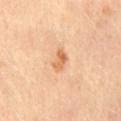  biopsy_status: not biopsied; imaged during a skin examination
  image:
    source: total-body photography crop
    field_of_view_mm: 15
  lesion_size:
    long_diameter_mm_approx: 3.0
  patient:
    sex: female
    age_approx: 50
  site: chest
  lighting: cross-polarized